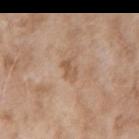Part of a total-body skin-imaging series; this lesion was reviewed on a skin check and was not flagged for biopsy.
Imaged with white-light lighting.
Automated tile analysis of the lesion measured a color-variation rating of about 2/10. And it measured an automated nevus-likeness rating near 0 out of 100 and a detector confidence of about 100 out of 100 that the crop contains a lesion.
A 15 mm close-up extracted from a 3D total-body photography capture.
A female patient in their mid-70s.
The lesion is located on the arm.